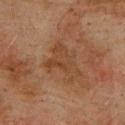Part of a total-body skin-imaging series; this lesion was reviewed on a skin check and was not flagged for biopsy. The subject is a male in their mid- to late 70s. Captured under cross-polarized illumination. A roughly 15 mm field-of-view crop from a total-body skin photograph. From the upper back. The lesion-visualizer software estimated a footprint of about 11 mm², an outline eccentricity of about 0.75 (0 = round, 1 = elongated), and a symmetry-axis asymmetry near 0.6. And it measured a lesion color around L≈35 a*≈18 b*≈28 in CIELAB, a lesion–skin lightness drop of about 5, and a lesion-to-skin contrast of about 5.5 (normalized; higher = more distinct).